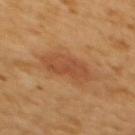Assessment: This lesion was catalogued during total-body skin photography and was not selected for biopsy. Background: A female patient, approximately 55 years of age. A lesion tile, about 15 mm wide, cut from a 3D total-body photograph. The lesion is on the upper back.